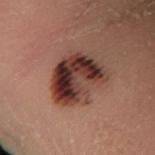Imaged during a routine full-body skin examination; the lesion was not biopsied and no histopathology is available. A lesion tile, about 15 mm wide, cut from a 3D total-body photograph. Imaged with cross-polarized lighting. The patient is a male about 65 years old. On the right forearm.